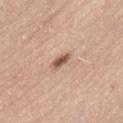Context: Imaged with white-light lighting. Cropped from a total-body skin-imaging series; the visible field is about 15 mm. Longest diameter approximately 2.5 mm. From the lower back. A male patient aged 63–67.